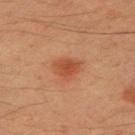The lesion was tiled from a total-body skin photograph and was not biopsied. An algorithmic analysis of the crop reported a shape eccentricity near 0.4 and two-axis asymmetry of about 0.25. The software also gave a lesion color around L≈45 a*≈26 b*≈35 in CIELAB and a normalized lesion–skin contrast near 7.5. The software also gave a border-irregularity index near 2.5/10 and a color-variation rating of about 2/10. A male subject, aged 33 to 37. About 2.5 mm across. Located on the right upper arm. A lesion tile, about 15 mm wide, cut from a 3D total-body photograph. Imaged with cross-polarized lighting.